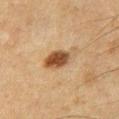Impression: Part of a total-body skin-imaging series; this lesion was reviewed on a skin check and was not flagged for biopsy. Acquisition and patient details: The lesion is on the chest. Captured under cross-polarized illumination. A male subject, roughly 50 years of age. Cropped from a whole-body photographic skin survey; the tile spans about 15 mm.